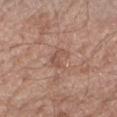Notes:
* illumination: white-light
* automated metrics: an area of roughly 4.5 mm² and an outline eccentricity of about 0.75 (0 = round, 1 = elongated); a lesion color around L≈53 a*≈20 b*≈27 in CIELAB, about 7 CIELAB-L* units darker than the surrounding skin, and a normalized lesion–skin contrast near 5
* location: the right forearm
* patient: male, about 60 years old
* image: 15 mm crop, total-body photography
* size: ≈3 mm